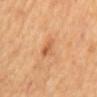  biopsy_status: not biopsied; imaged during a skin examination
  automated_metrics:
    area_mm2_approx: 4.5
    shape_asymmetry: 0.4
    cielab_L: 60
    cielab_a: 25
    cielab_b: 40
    vs_skin_darker_L: 9.0
    vs_skin_contrast_norm: 6.0
    nevus_likeness_0_100: 15
    lesion_detection_confidence_0_100: 100
  lesion_size:
    long_diameter_mm_approx: 3.0
  image:
    source: total-body photography crop
    field_of_view_mm: 15
  lighting: cross-polarized
  site: mid back
  patient:
    sex: male
    age_approx: 65